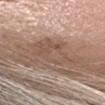<record>
<patient>
  <sex>female</sex>
  <age_approx>65</age_approx>
</patient>
<lighting>white-light</lighting>
<site>head or neck</site>
<image>
  <source>total-body photography crop</source>
  <field_of_view_mm>15</field_of_view_mm>
</image>
</record>